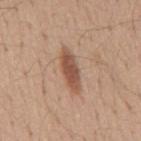The lesion was tiled from a total-body skin photograph and was not biopsied. A 15 mm crop from a total-body photograph taken for skin-cancer surveillance. Located on the back. The subject is a male aged around 55.